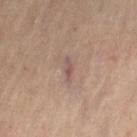Assessment: Part of a total-body skin-imaging series; this lesion was reviewed on a skin check and was not flagged for biopsy. Image and clinical context: A female patient, aged approximately 60. Located on the left thigh. Approximately 3 mm at its widest. Imaged with cross-polarized lighting. A roughly 15 mm field-of-view crop from a total-body skin photograph.